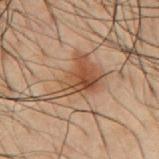biopsy_status: not biopsied; imaged during a skin examination
lighting: cross-polarized
image:
  source: total-body photography crop
  field_of_view_mm: 15
site: mid back
automated_metrics:
  vs_skin_darker_L: 9.0
  vs_skin_contrast_norm: 8.0
  border_irregularity_0_10: 7.0
  color_variation_0_10: 5.5
  peripheral_color_asymmetry: 2.0
patient:
  sex: male
  age_approx: 50